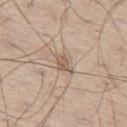Clinical impression:
Imaged during a routine full-body skin examination; the lesion was not biopsied and no histopathology is available.
Clinical summary:
Located on the left thigh. A 15 mm crop from a total-body photograph taken for skin-cancer surveillance. Imaged with white-light lighting. The lesion-visualizer software estimated an area of roughly 3.5 mm² and an outline eccentricity of about 0.8 (0 = round, 1 = elongated). The software also gave an automated nevus-likeness rating near 0 out of 100 and lesion-presence confidence of about 55/100. A male subject aged 58–62.